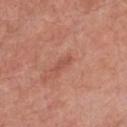Q: Was a biopsy performed?
A: catalogued during a skin exam; not biopsied
Q: What is the imaging modality?
A: ~15 mm tile from a whole-body skin photo
Q: How large is the lesion?
A: about 2.5 mm
Q: Lesion location?
A: the chest
Q: What are the patient's age and sex?
A: male, about 80 years old